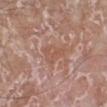workup: no biopsy performed (imaged during a skin exam)
illumination: white-light
patient: male, roughly 80 years of age
diameter: about 3.5 mm
anatomic site: the left lower leg
imaging modality: ~15 mm tile from a whole-body skin photo